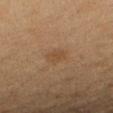Impression: Imaged during a routine full-body skin examination; the lesion was not biopsied and no histopathology is available. Background: Automated image analysis of the tile measured an area of roughly 4 mm² and a shape eccentricity near 0.7. Captured under cross-polarized illumination. On the right forearm. Cropped from a whole-body photographic skin survey; the tile spans about 15 mm. About 3 mm across. A female subject roughly 50 years of age.